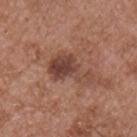{
  "biopsy_status": "not biopsied; imaged during a skin examination",
  "lesion_size": {
    "long_diameter_mm_approx": 5.5
  },
  "patient": {
    "sex": "male",
    "age_approx": 55
  },
  "site": "chest",
  "lighting": "white-light",
  "image": {
    "source": "total-body photography crop",
    "field_of_view_mm": 15
  }
}